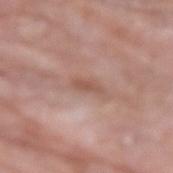Notes:
– notes · total-body-photography surveillance lesion; no biopsy
– image source · 15 mm crop, total-body photography
– patient · male, about 80 years old
– anatomic site · the right forearm
– tile lighting · white-light
– lesion size · about 2.5 mm